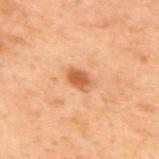The lesion was tiled from a total-body skin photograph and was not biopsied. Located on the upper back. The lesion's longest dimension is about 3 mm. Cropped from a whole-body photographic skin survey; the tile spans about 15 mm. A male patient aged approximately 70. Captured under cross-polarized illumination. Automated tile analysis of the lesion measured a footprint of about 4.5 mm², an eccentricity of roughly 0.8, and a shape-asymmetry score of about 0.25 (0 = symmetric). The software also gave a classifier nevus-likeness of about 80/100.